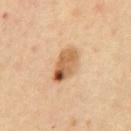{"biopsy_status": "not biopsied; imaged during a skin examination", "image": {"source": "total-body photography crop", "field_of_view_mm": 15}, "patient": {"sex": "female", "age_approx": 45}, "site": "abdomen", "lesion_size": {"long_diameter_mm_approx": 4.5}}